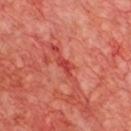The lesion was tiled from a total-body skin photograph and was not biopsied.
A male subject, aged around 65.
Longest diameter approximately 3 mm.
Cropped from a whole-body photographic skin survey; the tile spans about 15 mm.
The lesion is located on the chest.
This is a cross-polarized tile.
Automated image analysis of the tile measured an automated nevus-likeness rating near 0 out of 100 and lesion-presence confidence of about 70/100.